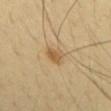Clinical impression:
Part of a total-body skin-imaging series; this lesion was reviewed on a skin check and was not flagged for biopsy.
Clinical summary:
Approximately 2.5 mm at its widest. Located on the back. A male patient about 45 years old. This image is a 15 mm lesion crop taken from a total-body photograph. Automated image analysis of the tile measured a footprint of about 4.5 mm², a shape eccentricity near 0.4, and a symmetry-axis asymmetry near 0.25. The software also gave an average lesion color of about L≈55 a*≈15 b*≈37 (CIELAB) and about 9 CIELAB-L* units darker than the surrounding skin. Imaged with cross-polarized lighting.